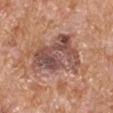Q: Is there a histopathology result?
A: imaged on a skin check; not biopsied
Q: What is the imaging modality?
A: 15 mm crop, total-body photography
Q: What lighting was used for the tile?
A: white-light illumination
Q: What is the anatomic site?
A: the right upper arm
Q: Lesion size?
A: ≈6.5 mm
Q: Who is the patient?
A: male, aged approximately 65
Q: What did automated image analysis measure?
A: an outline eccentricity of about 0.6 (0 = round, 1 = elongated)